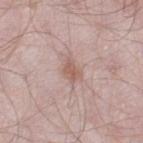No biopsy was performed on this lesion — it was imaged during a full skin examination and was not determined to be concerning. From the left thigh. A male patient, aged 53–57. This is a white-light tile. A roughly 15 mm field-of-view crop from a total-body skin photograph.